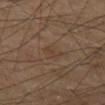Assessment: This lesion was catalogued during total-body skin photography and was not selected for biopsy. Context: The lesion is on the left thigh. A male subject, approximately 70 years of age. A region of skin cropped from a whole-body photographic capture, roughly 15 mm wide.